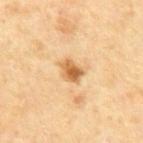Impression:
Recorded during total-body skin imaging; not selected for excision or biopsy.
Image and clinical context:
The lesion-visualizer software estimated an outline eccentricity of about 0.55 (0 = round, 1 = elongated) and two-axis asymmetry of about 0.3. The analysis additionally found border irregularity of about 2.5 on a 0–10 scale, a color-variation rating of about 4.5/10, and peripheral color asymmetry of about 1.5. The analysis additionally found an automated nevus-likeness rating near 85 out of 100. Imaged with cross-polarized lighting. From the abdomen. A male patient, aged around 65. Cropped from a whole-body photographic skin survey; the tile spans about 15 mm. About 2.5 mm across.